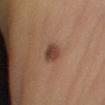Impression: Imaged during a routine full-body skin examination; the lesion was not biopsied and no histopathology is available.